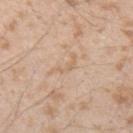The lesion was photographed on a routine skin check and not biopsied; there is no pathology result.
Automated image analysis of the tile measured a mean CIELAB color near L≈65 a*≈16 b*≈32, a lesion–skin lightness drop of about 6, and a normalized border contrast of about 4.5. It also reported internal color variation of about 0 on a 0–10 scale and a peripheral color-asymmetry measure near 0. And it measured an automated nevus-likeness rating near 0 out of 100 and lesion-presence confidence of about 95/100.
Captured under white-light illumination.
From the arm.
A 15 mm close-up tile from a total-body photography series done for melanoma screening.
A male subject about 25 years old.